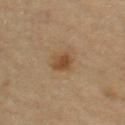Findings:
– follow-up: total-body-photography surveillance lesion; no biopsy
– patient: male, in their mid-80s or older
– site: the right upper arm
– lesion size: ~3 mm (longest diameter)
– tile lighting: cross-polarized
– image-analysis metrics: an average lesion color of about L≈45 a*≈17 b*≈33 (CIELAB), a lesion–skin lightness drop of about 9, and a lesion-to-skin contrast of about 8 (normalized; higher = more distinct); an automated nevus-likeness rating near 85 out of 100 and lesion-presence confidence of about 100/100
– imaging modality: 15 mm crop, total-body photography